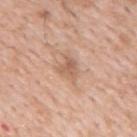Impression: Imaged during a routine full-body skin examination; the lesion was not biopsied and no histopathology is available. Acquisition and patient details: A male subject about 60 years old. Cropped from a total-body skin-imaging series; the visible field is about 15 mm. Captured under white-light illumination. The lesion is on the chest.